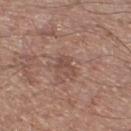Part of a total-body skin-imaging series; this lesion was reviewed on a skin check and was not flagged for biopsy. The subject is a male about 65 years old. The lesion is located on the left lower leg. The lesion-visualizer software estimated a classifier nevus-likeness of about 0/100 and lesion-presence confidence of about 100/100. A close-up tile cropped from a whole-body skin photograph, about 15 mm across. Approximately 2.5 mm at its widest.